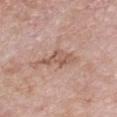On the chest. A female subject approximately 75 years of age. Cropped from a total-body skin-imaging series; the visible field is about 15 mm.